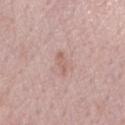Acquisition and patient details:
Imaged with white-light lighting. Approximately 2.5 mm at its widest. A close-up tile cropped from a whole-body skin photograph, about 15 mm across. The lesion-visualizer software estimated an eccentricity of roughly 0.9 and two-axis asymmetry of about 0.3. It also reported a mean CIELAB color near L≈60 a*≈20 b*≈25, a lesion–skin lightness drop of about 7, and a normalized lesion–skin contrast near 5.5. The analysis additionally found a classifier nevus-likeness of about 0/100. A male patient aged 53–57. On the right forearm.